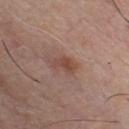Cropped from a total-body skin-imaging series; the visible field is about 15 mm.
Longest diameter approximately 3 mm.
The patient is a male roughly 55 years of age.
The lesion is on the chest.